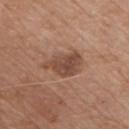Part of a total-body skin-imaging series; this lesion was reviewed on a skin check and was not flagged for biopsy. Captured under white-light illumination. Approximately 4.5 mm at its widest. A 15 mm crop from a total-body photograph taken for skin-cancer surveillance. Located on the chest. The patient is a male aged 73–77. The lesion-visualizer software estimated a footprint of about 9.5 mm², a shape eccentricity near 0.75, and a symmetry-axis asymmetry near 0.35. The analysis additionally found border irregularity of about 4 on a 0–10 scale and peripheral color asymmetry of about 1.